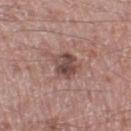workup: catalogued during a skin exam; not biopsied
body site: the left thigh
illumination: white-light
image: total-body-photography crop, ~15 mm field of view
diameter: ~3.5 mm (longest diameter)
patient: male, roughly 50 years of age